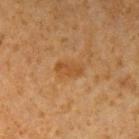anatomic site=the arm | acquisition=15 mm crop, total-body photography | lighting=cross-polarized | subject=male, aged 58–62 | lesion size=≈3.5 mm.